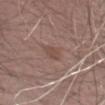This lesion was catalogued during total-body skin photography and was not selected for biopsy. Approximately 2.5 mm at its widest. A male patient aged approximately 65. The lesion is located on the left thigh. Captured under white-light illumination. Automated tile analysis of the lesion measured a footprint of about 3.5 mm², a shape eccentricity near 0.7, and two-axis asymmetry of about 0.3. It also reported an average lesion color of about L≈47 a*≈18 b*≈23 (CIELAB) and a normalized lesion–skin contrast near 5. The analysis additionally found a border-irregularity rating of about 2.5/10, a color-variation rating of about 1.5/10, and a peripheral color-asymmetry measure near 0.5. A close-up tile cropped from a whole-body skin photograph, about 15 mm across.